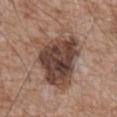{"biopsy_status": "not biopsied; imaged during a skin examination", "lighting": "white-light", "image": {"source": "total-body photography crop", "field_of_view_mm": 15}, "site": "mid back", "patient": {"sex": "male", "age_approx": 60}, "automated_metrics": {"vs_skin_darker_L": 14.0, "vs_skin_contrast_norm": 10.5, "lesion_detection_confidence_0_100": 100}}